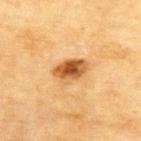The lesion is on the upper back. The patient is a female aged 78–82. This image is a 15 mm lesion crop taken from a total-body photograph. An algorithmic analysis of the crop reported a lesion area of about 7.5 mm², an eccentricity of roughly 0.8, and a symmetry-axis asymmetry near 0.15. And it measured a classifier nevus-likeness of about 95/100.